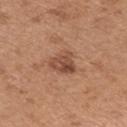This lesion was catalogued during total-body skin photography and was not selected for biopsy. A female patient, about 30 years old. The lesion is on the left upper arm. A 15 mm close-up tile from a total-body photography series done for melanoma screening.